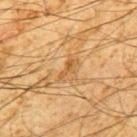workup: catalogued during a skin exam; not biopsied | location: the mid back | automated metrics: a mean CIELAB color near L≈48 a*≈19 b*≈37, about 7 CIELAB-L* units darker than the surrounding skin, and a lesion-to-skin contrast of about 6 (normalized; higher = more distinct); a border-irregularity rating of about 6/10, a color-variation rating of about 0/10, and peripheral color asymmetry of about 0; a classifier nevus-likeness of about 0/100 and a detector confidence of about 95 out of 100 that the crop contains a lesion | tile lighting: cross-polarized | patient: male, in their 60s | lesion diameter: ~3 mm (longest diameter) | image source: 15 mm crop, total-body photography.